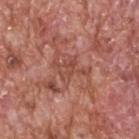Q: Is there a histopathology result?
A: imaged on a skin check; not biopsied
Q: What did automated image analysis measure?
A: an area of roughly 5.5 mm², an eccentricity of roughly 0.65, and a symmetry-axis asymmetry near 0.45; border irregularity of about 7.5 on a 0–10 scale, internal color variation of about 3 on a 0–10 scale, and peripheral color asymmetry of about 1
Q: What is the lesion's diameter?
A: ≈3.5 mm
Q: Illumination type?
A: white-light
Q: Who is the patient?
A: male, aged approximately 60
Q: How was this image acquired?
A: ~15 mm crop, total-body skin-cancer survey
Q: Lesion location?
A: the head or neck